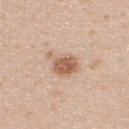- biopsy status: catalogued during a skin exam; not biopsied
- subject: male, aged 23 to 27
- anatomic site: the upper back
- imaging modality: ~15 mm tile from a whole-body skin photo